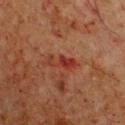| field | value |
|---|---|
| notes | no biopsy performed (imaged during a skin exam) |
| image source | ~15 mm crop, total-body skin-cancer survey |
| patient | male, in their mid-60s |
| diameter | ≈4 mm |
| illumination | cross-polarized illumination |
| site | the chest |